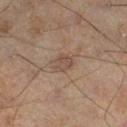Recorded during total-body skin imaging; not selected for excision or biopsy. The recorded lesion diameter is about 2.5 mm. A region of skin cropped from a whole-body photographic capture, roughly 15 mm wide. This is a cross-polarized tile. A male subject, in their mid-40s. The lesion-visualizer software estimated a normalized lesion–skin contrast near 5.5. The analysis additionally found a border-irregularity rating of about 2/10 and a peripheral color-asymmetry measure near 0.5. And it measured a classifier nevus-likeness of about 0/100. From the left lower leg.